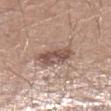Impression:
The lesion was tiled from a total-body skin photograph and was not biopsied.
Clinical summary:
The lesion is located on the left lower leg. Cropped from a total-body skin-imaging series; the visible field is about 15 mm. A male subject, aged approximately 30.